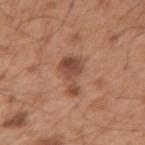| key | value |
|---|---|
| workup | no biopsy performed (imaged during a skin exam) |
| patient | male, aged approximately 55 |
| size | ~5 mm (longest diameter) |
| anatomic site | the right upper arm |
| TBP lesion metrics | roughly 11 lightness units darker than nearby skin and a lesion-to-skin contrast of about 8 (normalized; higher = more distinct) |
| acquisition | ~15 mm crop, total-body skin-cancer survey |